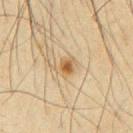Part of a total-body skin-imaging series; this lesion was reviewed on a skin check and was not flagged for biopsy.
A lesion tile, about 15 mm wide, cut from a 3D total-body photograph.
Captured under cross-polarized illumination.
From the chest.
A male subject, in their mid-60s.
The total-body-photography lesion software estimated a lesion area of about 5 mm², an outline eccentricity of about 0.65 (0 = round, 1 = elongated), and a symmetry-axis asymmetry near 0.35. The software also gave a within-lesion color-variation index near 5.5/10 and a peripheral color-asymmetry measure near 1.5. It also reported a classifier nevus-likeness of about 90/100 and lesion-presence confidence of about 100/100.